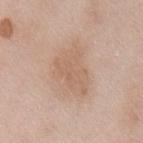Part of a total-body skin-imaging series; this lesion was reviewed on a skin check and was not flagged for biopsy. The patient is a male aged approximately 55. A close-up tile cropped from a whole-body skin photograph, about 15 mm across. On the front of the torso.